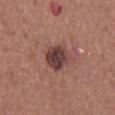Impression:
The lesion was tiled from a total-body skin photograph and was not biopsied.
Background:
Automated image analysis of the tile measured a lesion area of about 9 mm² and an outline eccentricity of about 0.7 (0 = round, 1 = elongated). The analysis additionally found a nevus-likeness score of about 0/100 and a lesion-detection confidence of about 100/100. A 15 mm close-up extracted from a 3D total-body photography capture. About 4 mm across. Imaged with white-light lighting. From the right thigh. A female patient, roughly 40 years of age.